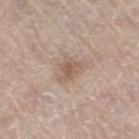Located on the leg.
A 15 mm crop from a total-body photograph taken for skin-cancer surveillance.
The tile uses white-light illumination.
Approximately 3 mm at its widest.
The subject is a female aged around 60.